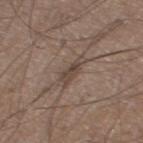No biopsy was performed on this lesion — it was imaged during a full skin examination and was not determined to be concerning. A male subject, aged approximately 20. About 4 mm across. Imaged with white-light lighting. A 15 mm close-up extracted from a 3D total-body photography capture. Located on the right thigh. Automated tile analysis of the lesion measured roughly 8 lightness units darker than nearby skin and a normalized lesion–skin contrast near 7. It also reported border irregularity of about 4 on a 0–10 scale and peripheral color asymmetry of about 1.5. It also reported a nevus-likeness score of about 0/100 and a lesion-detection confidence of about 55/100.